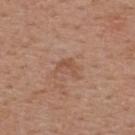Q: Was this lesion biopsied?
A: catalogued during a skin exam; not biopsied
Q: What did automated image analysis measure?
A: a mean CIELAB color near L≈52 a*≈20 b*≈30, roughly 6 lightness units darker than nearby skin, and a lesion-to-skin contrast of about 5 (normalized; higher = more distinct); internal color variation of about 2.5 on a 0–10 scale and a peripheral color-asymmetry measure near 1; a classifier nevus-likeness of about 0/100 and lesion-presence confidence of about 100/100
Q: How was this image acquired?
A: total-body-photography crop, ~15 mm field of view
Q: What are the patient's age and sex?
A: male, roughly 55 years of age
Q: How was the tile lit?
A: white-light
Q: What is the anatomic site?
A: the back
Q: How large is the lesion?
A: about 3 mm